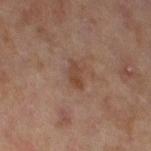Part of a total-body skin-imaging series; this lesion was reviewed on a skin check and was not flagged for biopsy. This image is a 15 mm lesion crop taken from a total-body photograph. Located on the leg. A female subject aged 58 to 62. About 2.5 mm across. Imaged with cross-polarized lighting. Automated image analysis of the tile measured an average lesion color of about L≈37 a*≈17 b*≈25 (CIELAB) and a normalized lesion–skin contrast near 6.5. The analysis additionally found border irregularity of about 3 on a 0–10 scale, a within-lesion color-variation index near 0.5/10, and radial color variation of about 0. The analysis additionally found a classifier nevus-likeness of about 5/100 and a detector confidence of about 100 out of 100 that the crop contains a lesion.